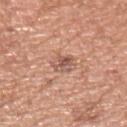* patient · male, aged 63 to 67
* image-analysis metrics · an area of roughly 5 mm² and a shape-asymmetry score of about 0.25 (0 = symmetric); a classifier nevus-likeness of about 0/100 and lesion-presence confidence of about 100/100
* anatomic site · the left upper arm
* acquisition · ~15 mm crop, total-body skin-cancer survey